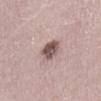Imaged during a routine full-body skin examination; the lesion was not biopsied and no histopathology is available. Located on the abdomen. The recorded lesion diameter is about 3.5 mm. A 15 mm close-up tile from a total-body photography series done for melanoma screening. This is a white-light tile. A male subject, about 50 years old.